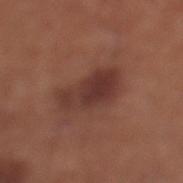• image-analysis metrics · an automated nevus-likeness rating near 85 out of 100
• location · the left lower leg
• acquisition · total-body-photography crop, ~15 mm field of view
• patient · male, aged around 70
• size · ≈6 mm
• lighting · white-light illumination